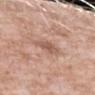workup: total-body-photography surveillance lesion; no biopsy | size: ~3 mm (longest diameter) | patient: female, approximately 70 years of age | image: ~15 mm crop, total-body skin-cancer survey | location: the arm | TBP lesion metrics: a footprint of about 5 mm², an outline eccentricity of about 0.75 (0 = round, 1 = elongated), and a symmetry-axis asymmetry near 0.25; a mean CIELAB color near L≈57 a*≈21 b*≈27, roughly 10 lightness units darker than nearby skin, and a normalized border contrast of about 6.5; a border-irregularity rating of about 2.5/10, a color-variation rating of about 2/10, and peripheral color asymmetry of about 0.5 | illumination: white-light illumination.